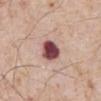follow-up: catalogued during a skin exam; not biopsied
subject: male, roughly 80 years of age
imaging modality: ~15 mm crop, total-body skin-cancer survey
size: ~3.5 mm (longest diameter)
tile lighting: white-light illumination
anatomic site: the front of the torso
TBP lesion metrics: a lesion color around L≈47 a*≈27 b*≈19 in CIELAB and a normalized lesion–skin contrast near 15; internal color variation of about 6 on a 0–10 scale and peripheral color asymmetry of about 2